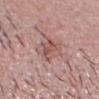Clinical impression: Captured during whole-body skin photography for melanoma surveillance; the lesion was not biopsied. Image and clinical context: This image is a 15 mm lesion crop taken from a total-body photograph. The tile uses white-light illumination. The recorded lesion diameter is about 4.5 mm. A male patient, roughly 30 years of age. The lesion-visualizer software estimated about 9 CIELAB-L* units darker than the surrounding skin and a lesion-to-skin contrast of about 6 (normalized; higher = more distinct). It also reported a nevus-likeness score of about 0/100 and lesion-presence confidence of about 100/100. Located on the head or neck.